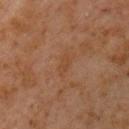Imaged during a routine full-body skin examination; the lesion was not biopsied and no histopathology is available.